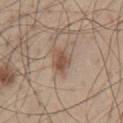Imaged during a routine full-body skin examination; the lesion was not biopsied and no histopathology is available. Cropped from a whole-body photographic skin survey; the tile spans about 15 mm. Imaged with white-light lighting. The lesion is on the chest. A male subject aged 43 to 47.